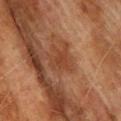{"biopsy_status": "not biopsied; imaged during a skin examination", "site": "back", "patient": {"sex": "male", "age_approx": 75}, "image": {"source": "total-body photography crop", "field_of_view_mm": 15}}